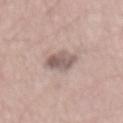Part of a total-body skin-imaging series; this lesion was reviewed on a skin check and was not flagged for biopsy.
A male patient, in their 40s.
From the lower back.
The lesion's longest dimension is about 3.5 mm.
A region of skin cropped from a whole-body photographic capture, roughly 15 mm wide.
Captured under white-light illumination.